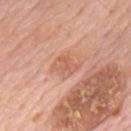{
  "biopsy_status": "not biopsied; imaged during a skin examination",
  "patient": {
    "sex": "male",
    "age_approx": 80
  },
  "lesion_size": {
    "long_diameter_mm_approx": 3.0
  },
  "lighting": "white-light",
  "image": {
    "source": "total-body photography crop",
    "field_of_view_mm": 15
  },
  "site": "mid back"
}